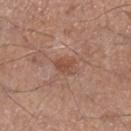Clinical impression:
Captured during whole-body skin photography for melanoma surveillance; the lesion was not biopsied.
Background:
From the leg. The lesion's longest dimension is about 2.5 mm. A male patient aged 53–57. The tile uses white-light illumination. This image is a 15 mm lesion crop taken from a total-body photograph.